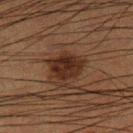The lesion was photographed on a routine skin check and not biopsied; there is no pathology result. Located on the left lower leg. A male subject about 50 years old. The recorded lesion diameter is about 4 mm. The tile uses cross-polarized illumination. A 15 mm close-up tile from a total-body photography series done for melanoma screening.